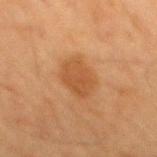Recorded during total-body skin imaging; not selected for excision or biopsy. The lesion is on the mid back. A male patient, about 65 years old. Measured at roughly 4.5 mm in maximum diameter. A lesion tile, about 15 mm wide, cut from a 3D total-body photograph. The tile uses cross-polarized illumination.